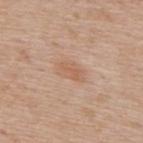Q: Was this lesion biopsied?
A: catalogued during a skin exam; not biopsied
Q: Patient demographics?
A: male, roughly 60 years of age
Q: How was this image acquired?
A: total-body-photography crop, ~15 mm field of view
Q: What is the lesion's diameter?
A: ≈3.5 mm
Q: Lesion location?
A: the upper back
Q: Automated lesion metrics?
A: a lesion–skin lightness drop of about 7 and a normalized border contrast of about 5